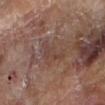  biopsy_status: not biopsied; imaged during a skin examination
  patient:
    sex: male
    age_approx: 85+
  automated_metrics:
    cielab_L: 40
    cielab_a: 17
    cielab_b: 22
    vs_skin_darker_L: 5.0
    nevus_likeness_0_100: 0
    lesion_detection_confidence_0_100: 55
  image:
    source: total-body photography crop
    field_of_view_mm: 15
  lesion_size:
    long_diameter_mm_approx: 4.5
  site: right forearm